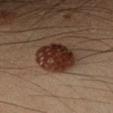Recorded during total-body skin imaging; not selected for excision or biopsy. A male patient, roughly 35 years of age. The total-body-photography lesion software estimated a border-irregularity index near 1.5/10, internal color variation of about 6 on a 0–10 scale, and peripheral color asymmetry of about 2. A region of skin cropped from a whole-body photographic capture, roughly 15 mm wide. The lesion is on the arm.